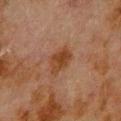Q: Is there a histopathology result?
A: total-body-photography surveillance lesion; no biopsy
Q: Patient demographics?
A: male, approximately 80 years of age
Q: How large is the lesion?
A: ~3.5 mm (longest diameter)
Q: What is the imaging modality?
A: ~15 mm crop, total-body skin-cancer survey
Q: What lighting was used for the tile?
A: cross-polarized illumination
Q: Where on the body is the lesion?
A: the upper back